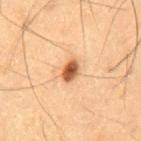Clinical impression: The lesion was photographed on a routine skin check and not biopsied; there is no pathology result. Background: Cropped from a total-body skin-imaging series; the visible field is about 15 mm. A male subject, about 60 years old. Longest diameter approximately 3 mm. This is a cross-polarized tile.